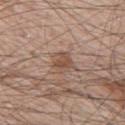Assessment: The lesion was tiled from a total-body skin photograph and was not biopsied. Clinical summary: On the right upper arm. An algorithmic analysis of the crop reported a lesion area of about 5.5 mm² and a symmetry-axis asymmetry near 0.25. And it measured an automated nevus-likeness rating near 0 out of 100 and lesion-presence confidence of about 100/100. The tile uses white-light illumination. The subject is a male in their mid-60s. Cropped from a whole-body photographic skin survey; the tile spans about 15 mm. The lesion's longest dimension is about 3 mm.